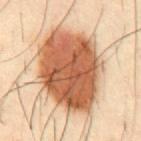• anatomic site — the abdomen
• subject — male, approximately 40 years of age
• lesion diameter — ≈9.5 mm
• illumination — cross-polarized illumination
• acquisition — ~15 mm tile from a whole-body skin photo